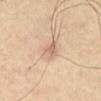Impression:
The lesion was tiled from a total-body skin photograph and was not biopsied.
Clinical summary:
Captured under cross-polarized illumination. A 15 mm crop from a total-body photograph taken for skin-cancer surveillance. An algorithmic analysis of the crop reported an area of roughly 5 mm², an eccentricity of roughly 0.85, and two-axis asymmetry of about 0.3. It also reported a border-irregularity index near 3/10, internal color variation of about 3.5 on a 0–10 scale, and a peripheral color-asymmetry measure near 1.5. The analysis additionally found a classifier nevus-likeness of about 0/100 and a lesion-detection confidence of about 80/100. Approximately 3.5 mm at its widest. From the abdomen.